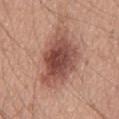notes: no biopsy performed (imaged during a skin exam) | patient: male, aged approximately 60 | image source: ~15 mm tile from a whole-body skin photo | lesion size: about 8.5 mm | lighting: white-light illumination | body site: the abdomen.